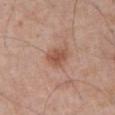| feature | finding |
|---|---|
| tile lighting | white-light illumination |
| site | the front of the torso |
| image source | total-body-photography crop, ~15 mm field of view |
| patient | male, aged around 70 |
| lesion size | ≈3 mm |